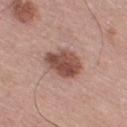<record>
  <biopsy_status>not biopsied; imaged during a skin examination</biopsy_status>
  <lesion_size>
    <long_diameter_mm_approx>4.0</long_diameter_mm_approx>
  </lesion_size>
  <site>right thigh</site>
  <patient>
    <sex>male</sex>
    <age_approx>70</age_approx>
  </patient>
  <image>
    <source>total-body photography crop</source>
    <field_of_view_mm>15</field_of_view_mm>
  </image>
  <automated_metrics>
    <area_mm2_approx>12.0</area_mm2_approx>
    <shape_asymmetry>0.2</shape_asymmetry>
    <cielab_L>49</cielab_L>
    <cielab_a>22</cielab_a>
    <cielab_b>26</cielab_b>
    <vs_skin_darker_L>14.0</vs_skin_darker_L>
    <vs_skin_contrast_norm>10.0</vs_skin_contrast_norm>
    <color_variation_0_10>4.5</color_variation_0_10>
  </automated_metrics>
</record>